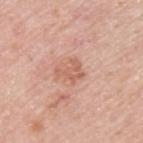Impression:
No biopsy was performed on this lesion — it was imaged during a full skin examination and was not determined to be concerning.
Clinical summary:
From the back. Cropped from a whole-body photographic skin survey; the tile spans about 15 mm. A male subject, aged approximately 45. Imaged with white-light lighting.